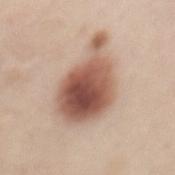workup — no biopsy performed (imaged during a skin exam)
patient — female, aged around 50
image source — 15 mm crop, total-body photography
location — the mid back